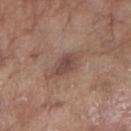Q: Was a biopsy performed?
A: catalogued during a skin exam; not biopsied
Q: Lesion location?
A: the left forearm
Q: What kind of image is this?
A: ~15 mm tile from a whole-body skin photo
Q: Illumination type?
A: white-light
Q: What did automated image analysis measure?
A: a lesion–skin lightness drop of about 9; a border-irregularity rating of about 2/10, a within-lesion color-variation index near 2.5/10, and a peripheral color-asymmetry measure near 1; a detector confidence of about 100 out of 100 that the crop contains a lesion
Q: Patient demographics?
A: female, aged 73 to 77
Q: How large is the lesion?
A: ≈4 mm